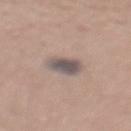  site: mid back
  patient:
    sex: male
    age_approx: 45
  image:
    source: total-body photography crop
    field_of_view_mm: 15